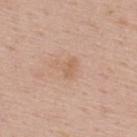notes: imaged on a skin check; not biopsied | lesion size: ~2.5 mm (longest diameter) | TBP lesion metrics: a footprint of about 4 mm²; a lesion color around L≈62 a*≈19 b*≈31 in CIELAB and a normalized border contrast of about 4.5 | acquisition: ~15 mm crop, total-body skin-cancer survey | body site: the upper back | patient: male, approximately 55 years of age.